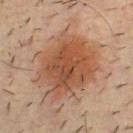The lesion was tiled from a total-body skin photograph and was not biopsied. An algorithmic analysis of the crop reported roughly 10 lightness units darker than nearby skin and a lesion-to-skin contrast of about 8.5 (normalized; higher = more distinct). The software also gave a border-irregularity index near 3/10 and a within-lesion color-variation index near 6/10. On the chest. Imaged with cross-polarized lighting. Longest diameter approximately 8 mm. Cropped from a total-body skin-imaging series; the visible field is about 15 mm. The subject is a male aged 33–37.